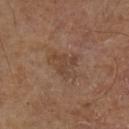Captured during whole-body skin photography for melanoma surveillance; the lesion was not biopsied.
A roughly 15 mm field-of-view crop from a total-body skin photograph.
This is a cross-polarized tile.
A male subject, in their mid- to late 60s.
Automated image analysis of the tile measured a lesion color around L≈41 a*≈16 b*≈26 in CIELAB, about 6 CIELAB-L* units darker than the surrounding skin, and a lesion-to-skin contrast of about 5 (normalized; higher = more distinct). And it measured a border-irregularity rating of about 4/10, internal color variation of about 3 on a 0–10 scale, and radial color variation of about 1. The analysis additionally found a detector confidence of about 100 out of 100 that the crop contains a lesion.
Measured at roughly 4 mm in maximum diameter.